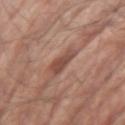Part of a total-body skin-imaging series; this lesion was reviewed on a skin check and was not flagged for biopsy. On the left upper arm. The total-body-photography lesion software estimated a footprint of about 5.5 mm², an eccentricity of roughly 0.85, and two-axis asymmetry of about 0.35. The software also gave a lesion color around L≈48 a*≈21 b*≈26 in CIELAB, a lesion–skin lightness drop of about 10, and a normalized lesion–skin contrast near 7.5. And it measured a border-irregularity rating of about 3.5/10, internal color variation of about 2.5 on a 0–10 scale, and peripheral color asymmetry of about 0.5. It also reported an automated nevus-likeness rating near 0 out of 100 and a lesion-detection confidence of about 100/100. This image is a 15 mm lesion crop taken from a total-body photograph. This is a white-light tile. Approximately 3.5 mm at its widest. A male patient aged approximately 65.